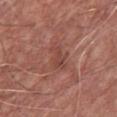| key | value |
|---|---|
| notes | imaged on a skin check; not biopsied |
| subject | male, roughly 75 years of age |
| anatomic site | the chest |
| lighting | white-light illumination |
| lesion size | ≈3 mm |
| image | ~15 mm crop, total-body skin-cancer survey |